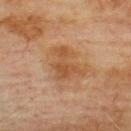No biopsy was performed on this lesion — it was imaged during a full skin examination and was not determined to be concerning.
A roughly 15 mm field-of-view crop from a total-body skin photograph.
Located on the back.
A male subject roughly 75 years of age.
The tile uses cross-polarized illumination.